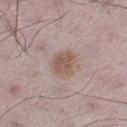Notes:
* biopsy status: catalogued during a skin exam; not biopsied
* body site: the right lower leg
* imaging modality: total-body-photography crop, ~15 mm field of view
* subject: male, aged approximately 50
* diameter: ~3 mm (longest diameter)
* illumination: white-light illumination